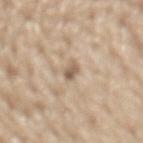Clinical impression: Imaged during a routine full-body skin examination; the lesion was not biopsied and no histopathology is available. Background: The lesion is located on the back. Cropped from a total-body skin-imaging series; the visible field is about 15 mm. About 2.5 mm across. The patient is a male in their 70s. Automated tile analysis of the lesion measured a mean CIELAB color near L≈59 a*≈14 b*≈28, roughly 12 lightness units darker than nearby skin, and a lesion-to-skin contrast of about 7.5 (normalized; higher = more distinct). And it measured a border-irregularity rating of about 3/10, a within-lesion color-variation index near 3/10, and a peripheral color-asymmetry measure near 1.5. Captured under white-light illumination.